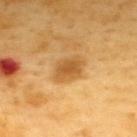Recorded during total-body skin imaging; not selected for excision or biopsy.
Located on the upper back.
This image is a 15 mm lesion crop taken from a total-body photograph.
This is a cross-polarized tile.
Longest diameter approximately 3.5 mm.
The subject is a male aged around 60.